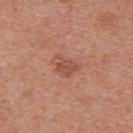Impression:
This lesion was catalogued during total-body skin photography and was not selected for biopsy.
Acquisition and patient details:
A female patient, aged 38 to 42. A 15 mm close-up tile from a total-body photography series done for melanoma screening. The recorded lesion diameter is about 3.5 mm. From the upper back. Captured under white-light illumination.